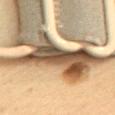Clinical impression: This lesion was catalogued during total-body skin photography and was not selected for biopsy. Context: The lesion is on the mid back. A female subject, approximately 55 years of age. The lesion's longest dimension is about 13.5 mm. The tile uses cross-polarized illumination. A close-up tile cropped from a whole-body skin photograph, about 15 mm across.